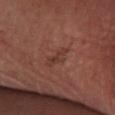| key | value |
|---|---|
| biopsy status | imaged on a skin check; not biopsied |
| location | the left lower leg |
| subject | male, about 65 years old |
| imaging modality | ~15 mm tile from a whole-body skin photo |
| lesion diameter | ≈4 mm |
| illumination | cross-polarized illumination |